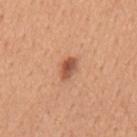Assessment: The lesion was tiled from a total-body skin photograph and was not biopsied. Context: A 15 mm crop from a total-body photograph taken for skin-cancer surveillance. The lesion is located on the back. Captured under white-light illumination. The recorded lesion diameter is about 3 mm. Automated tile analysis of the lesion measured a lesion area of about 4 mm², an outline eccentricity of about 0.8 (0 = round, 1 = elongated), and a shape-asymmetry score of about 0.2 (0 = symmetric). The software also gave a lesion color around L≈54 a*≈26 b*≈33 in CIELAB, about 13 CIELAB-L* units darker than the surrounding skin, and a normalized lesion–skin contrast near 8.5. And it measured a classifier nevus-likeness of about 95/100 and lesion-presence confidence of about 100/100. A male patient aged approximately 55.